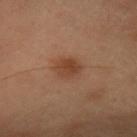The lesion was tiled from a total-body skin photograph and was not biopsied. Cropped from a whole-body photographic skin survey; the tile spans about 15 mm. A female patient, aged 53 to 57. An algorithmic analysis of the crop reported a border-irregularity rating of about 1.5/10, internal color variation of about 2.5 on a 0–10 scale, and a peripheral color-asymmetry measure near 1. And it measured an automated nevus-likeness rating near 90 out of 100. The lesion's longest dimension is about 3.5 mm. The tile uses cross-polarized illumination. From the head or neck.